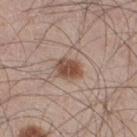The lesion was tiled from a total-body skin photograph and was not biopsied.